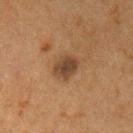body site = the left upper arm | subject = female, aged 58 to 62 | image = 15 mm crop, total-body photography.